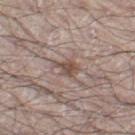{"biopsy_status": "not biopsied; imaged during a skin examination", "lighting": "white-light", "patient": {"sex": "male", "age_approx": 70}, "site": "right leg", "automated_metrics": {"color_variation_0_10": 2.5}, "lesion_size": {"long_diameter_mm_approx": 3.0}, "image": {"source": "total-body photography crop", "field_of_view_mm": 15}}